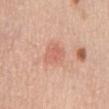Impression:
The lesion was photographed on a routine skin check and not biopsied; there is no pathology result.
Background:
The total-body-photography lesion software estimated an area of roughly 7 mm² and a shape eccentricity near 0.35. And it measured a mean CIELAB color near L≈63 a*≈26 b*≈30 and a lesion-to-skin contrast of about 5 (normalized; higher = more distinct). A female patient aged 63 to 67. The tile uses white-light illumination. Cropped from a whole-body photographic skin survey; the tile spans about 15 mm. Located on the chest. Measured at roughly 3 mm in maximum diameter.